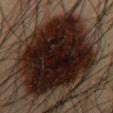Assessment:
This lesion was catalogued during total-body skin photography and was not selected for biopsy.
Context:
Located on the abdomen. A close-up tile cropped from a whole-body skin photograph, about 15 mm across. The subject is a male approximately 55 years of age.